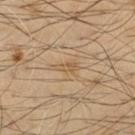| key | value |
|---|---|
| follow-up | total-body-photography surveillance lesion; no biopsy |
| location | the chest |
| image source | ~15 mm tile from a whole-body skin photo |
| tile lighting | cross-polarized |
| subject | male, in their mid-50s |
| size | ~2.5 mm (longest diameter) |